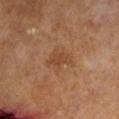| feature | finding |
|---|---|
| workup | total-body-photography surveillance lesion; no biopsy |
| size | about 3.5 mm |
| acquisition | ~15 mm crop, total-body skin-cancer survey |
| subject | male, approximately 65 years of age |
| site | the left lower leg |